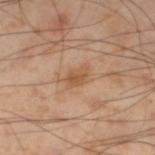biopsy status — total-body-photography surveillance lesion; no biopsy | TBP lesion metrics — an area of roughly 4 mm², an eccentricity of roughly 0.8, and two-axis asymmetry of about 0.2; an average lesion color of about L≈54 a*≈21 b*≈34 (CIELAB), about 8 CIELAB-L* units darker than the surrounding skin, and a normalized border contrast of about 6.5 | patient — male, aged approximately 55 | imaging modality — ~15 mm crop, total-body skin-cancer survey | lesion diameter — about 3 mm | anatomic site — the leg.